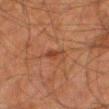Case summary:
• follow-up — imaged on a skin check; not biopsied
• subject — male, in their 80s
• site — the right thigh
• image — total-body-photography crop, ~15 mm field of view
• diameter — ≈2.5 mm